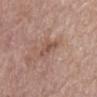workup: total-body-photography surveillance lesion; no biopsy | illumination: white-light illumination | image-analysis metrics: a footprint of about 4.5 mm², an eccentricity of roughly 0.9, and a shape-asymmetry score of about 0.4 (0 = symmetric); a mean CIELAB color near L≈51 a*≈20 b*≈26, a lesion–skin lightness drop of about 8, and a normalized lesion–skin contrast near 6.5; a peripheral color-asymmetry measure near 0.5; a nevus-likeness score of about 0/100 and a detector confidence of about 100 out of 100 that the crop contains a lesion | acquisition: ~15 mm crop, total-body skin-cancer survey | anatomic site: the abdomen | subject: female, in their mid- to late 70s.